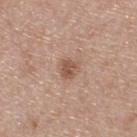Assessment:
Captured during whole-body skin photography for melanoma surveillance; the lesion was not biopsied.
Context:
Captured under white-light illumination. A 15 mm close-up extracted from a 3D total-body photography capture. A male subject, aged 53 to 57. The lesion-visualizer software estimated an average lesion color of about L≈54 a*≈20 b*≈28 (CIELAB), roughly 10 lightness units darker than nearby skin, and a normalized border contrast of about 7. And it measured a color-variation rating of about 2/10 and radial color variation of about 1. The software also gave a classifier nevus-likeness of about 65/100 and a lesion-detection confidence of about 100/100. Approximately 2.5 mm at its widest.